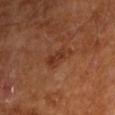Case summary:
- follow-up — total-body-photography surveillance lesion; no biopsy
- location — the arm
- tile lighting — cross-polarized
- diameter — about 3.5 mm
- image source — ~15 mm tile from a whole-body skin photo
- patient — male, roughly 65 years of age